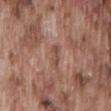Clinical impression: This lesion was catalogued during total-body skin photography and was not selected for biopsy. Context: A male subject about 75 years old. Imaged with white-light lighting. From the lower back. Cropped from a total-body skin-imaging series; the visible field is about 15 mm.